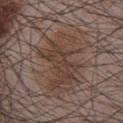This lesion was catalogued during total-body skin photography and was not selected for biopsy.
From the chest.
A male patient aged 63 to 67.
Imaged with white-light lighting.
Approximately 7 mm at its widest.
A region of skin cropped from a whole-body photographic capture, roughly 15 mm wide.